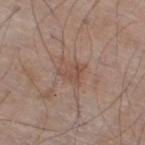Q: Was a biopsy performed?
A: imaged on a skin check; not biopsied
Q: Who is the patient?
A: male, approximately 70 years of age
Q: What is the anatomic site?
A: the right thigh
Q: What is the imaging modality?
A: 15 mm crop, total-body photography